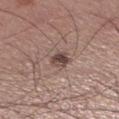diameter = about 2.5 mm | tile lighting = white-light illumination | subject = male, roughly 55 years of age | image = total-body-photography crop, ~15 mm field of view | site = the right lower leg | automated lesion analysis = a footprint of about 4 mm², an eccentricity of roughly 0.45, and two-axis asymmetry of about 0.25; border irregularity of about 2 on a 0–10 scale, internal color variation of about 4.5 on a 0–10 scale, and a peripheral color-asymmetry measure near 1.5; an automated nevus-likeness rating near 90 out of 100 and a detector confidence of about 100 out of 100 that the crop contains a lesion.